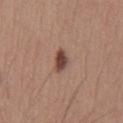Part of a total-body skin-imaging series; this lesion was reviewed on a skin check and was not flagged for biopsy. The lesion is located on the chest. A region of skin cropped from a whole-body photographic capture, roughly 15 mm wide. The tile uses white-light illumination. A male patient, approximately 35 years of age. Approximately 3 mm at its widest.